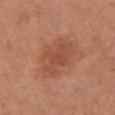Assessment: The lesion was photographed on a routine skin check and not biopsied; there is no pathology result. Acquisition and patient details: The lesion is on the front of the torso. Cropped from a total-body skin-imaging series; the visible field is about 15 mm. The patient is a female aged approximately 65. The lesion's longest dimension is about 5.5 mm.